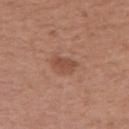| field | value |
|---|---|
| follow-up | catalogued during a skin exam; not biopsied |
| diameter | about 3 mm |
| body site | the right upper arm |
| imaging modality | 15 mm crop, total-body photography |
| subject | male, in their mid- to late 50s |